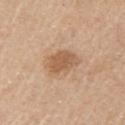No biopsy was performed on this lesion — it was imaged during a full skin examination and was not determined to be concerning.
The lesion-visualizer software estimated a footprint of about 9.5 mm², an outline eccentricity of about 0.6 (0 = round, 1 = elongated), and a symmetry-axis asymmetry near 0.25. It also reported a lesion-detection confidence of about 100/100.
On the right upper arm.
Longest diameter approximately 4 mm.
This image is a 15 mm lesion crop taken from a total-body photograph.
The subject is a male aged 63 to 67.